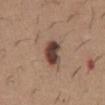workup = no biopsy performed (imaged during a skin exam) | automated lesion analysis = an area of roughly 7.5 mm² and a symmetry-axis asymmetry near 0.2; a border-irregularity rating of about 2/10 and a within-lesion color-variation index near 4.5/10 | subject = male, in their 60s | acquisition = total-body-photography crop, ~15 mm field of view | size = ~3.5 mm (longest diameter) | tile lighting = white-light illumination | anatomic site = the abdomen.